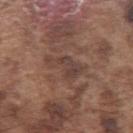| feature | finding |
|---|---|
| notes | no biopsy performed (imaged during a skin exam) |
| anatomic site | the left upper arm |
| patient | male, aged approximately 75 |
| tile lighting | white-light illumination |
| automated lesion analysis | a mean CIELAB color near L≈41 a*≈17 b*≈22, a lesion–skin lightness drop of about 7, and a normalized border contrast of about 6.5; a border-irregularity rating of about 6.5/10, internal color variation of about 2.5 on a 0–10 scale, and peripheral color asymmetry of about 1 |
| lesion diameter | ~4.5 mm (longest diameter) |
| image source | total-body-photography crop, ~15 mm field of view |